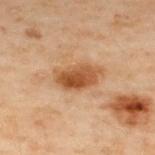Case summary:
– notes: catalogued during a skin exam; not biopsied
– patient: male, aged around 50
– tile lighting: cross-polarized
– location: the upper back
– image: ~15 mm crop, total-body skin-cancer survey
– automated metrics: a footprint of about 11 mm² and an outline eccentricity of about 0.85 (0 = round, 1 = elongated); a border-irregularity rating of about 2.5/10 and internal color variation of about 5 on a 0–10 scale; lesion-presence confidence of about 100/100